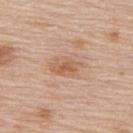tile lighting=white-light
size=about 4.5 mm
anatomic site=the upper back
image source=~15 mm tile from a whole-body skin photo
patient=male, roughly 80 years of age